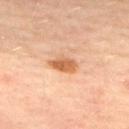Acquisition and patient details:
A female patient aged 48 to 52. The lesion is located on the chest. Cropped from a total-body skin-imaging series; the visible field is about 15 mm. This is a cross-polarized tile. Longest diameter approximately 3.5 mm.